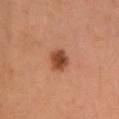{"biopsy_status": "not biopsied; imaged during a skin examination", "lighting": "cross-polarized", "patient": {"sex": "female", "age_approx": 55}, "image": {"source": "total-body photography crop", "field_of_view_mm": 15}, "site": "head or neck", "automated_metrics": {"border_irregularity_0_10": 1.5, "color_variation_0_10": 3.5, "peripheral_color_asymmetry": 1.0, "nevus_likeness_0_100": 100, "lesion_detection_confidence_0_100": 100}}